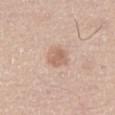workup — total-body-photography surveillance lesion; no biopsy
body site — the left thigh
illumination — white-light
acquisition — 15 mm crop, total-body photography
subject — male, aged around 65
lesion diameter — about 3 mm
automated metrics — a border-irregularity rating of about 1.5/10 and internal color variation of about 3 on a 0–10 scale